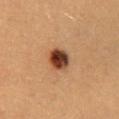Assessment:
No biopsy was performed on this lesion — it was imaged during a full skin examination and was not determined to be concerning.
Context:
A male subject, roughly 20 years of age. A 15 mm close-up extracted from a 3D total-body photography capture. Automated tile analysis of the lesion measured a mean CIELAB color near L≈40 a*≈23 b*≈32, about 19 CIELAB-L* units darker than the surrounding skin, and a normalized lesion–skin contrast near 14.5. The analysis additionally found a border-irregularity rating of about 1.5/10, a color-variation rating of about 7.5/10, and radial color variation of about 2.5. And it measured an automated nevus-likeness rating near 100 out of 100 and a lesion-detection confidence of about 100/100. Measured at roughly 3 mm in maximum diameter. Captured under cross-polarized illumination. Located on the lower back.